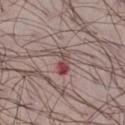Part of a total-body skin-imaging series; this lesion was reviewed on a skin check and was not flagged for biopsy. Longest diameter approximately 3.5 mm. The lesion is on the right thigh. A male patient aged 38–42. A roughly 15 mm field-of-view crop from a total-body skin photograph. The tile uses white-light illumination. The total-body-photography lesion software estimated a lesion area of about 4 mm² and a shape eccentricity near 0.85.